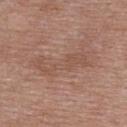follow-up = total-body-photography surveillance lesion; no biopsy | image source = total-body-photography crop, ~15 mm field of view | subject = female, approximately 50 years of age | site = the back | TBP lesion metrics = about 6 CIELAB-L* units darker than the surrounding skin and a normalized border contrast of about 4.5; border irregularity of about 8 on a 0–10 scale and radial color variation of about 0.5; an automated nevus-likeness rating near 0 out of 100 | lesion size = ≈7.5 mm | lighting = white-light illumination.